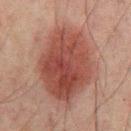<lesion>
  <biopsy_status>not biopsied; imaged during a skin examination</biopsy_status>
  <site>mid back</site>
  <lesion_size>
    <long_diameter_mm_approx>9.0</long_diameter_mm_approx>
  </lesion_size>
  <lighting>cross-polarized</lighting>
  <patient>
    <sex>male</sex>
    <age_approx>65</age_approx>
  </patient>
  <image>
    <source>total-body photography crop</source>
    <field_of_view_mm>15</field_of_view_mm>
  </image>
  <automated_metrics>
    <area_mm2_approx>42.0</area_mm2_approx>
    <shape_asymmetry>0.1</shape_asymmetry>
    <cielab_L>37</cielab_L>
    <cielab_a>21</cielab_a>
    <cielab_b>22</cielab_b>
    <vs_skin_darker_L>11.0</vs_skin_darker_L>
    <vs_skin_contrast_norm>9.5</vs_skin_contrast_norm>
    <border_irregularity_0_10>2.0</border_irregularity_0_10>
    <color_variation_0_10>5.0</color_variation_0_10>
    <peripheral_color_asymmetry>2.0</peripheral_color_asymmetry>
  </automated_metrics>
</lesion>